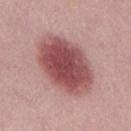{
  "biopsy_status": "not biopsied; imaged during a skin examination",
  "lesion_size": {
    "long_diameter_mm_approx": 8.5
  },
  "image": {
    "source": "total-body photography crop",
    "field_of_view_mm": 15
  },
  "patient": {
    "sex": "male",
    "age_approx": 30
  },
  "lighting": "white-light",
  "automated_metrics": {
    "vs_skin_contrast_norm": 10.5,
    "border_irregularity_0_10": 1.5,
    "color_variation_0_10": 5.0,
    "peripheral_color_asymmetry": 1.0
  }
}